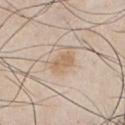<record>
<biopsy_status>not biopsied; imaged during a skin examination</biopsy_status>
<automated_metrics>
  <area_mm2_approx>6.0</area_mm2_approx>
  <eccentricity>0.8</eccentricity>
  <shape_asymmetry>0.2</shape_asymmetry>
  <cielab_L>64</cielab_L>
  <cielab_a>15</cielab_a>
  <cielab_b>32</cielab_b>
  <vs_skin_contrast_norm>6.5</vs_skin_contrast_norm>
  <nevus_likeness_0_100>45</nevus_likeness_0_100>
  <lesion_detection_confidence_0_100>100</lesion_detection_confidence_0_100>
</automated_metrics>
<patient>
  <sex>male</sex>
  <age_approx>45</age_approx>
</patient>
<image>
  <source>total-body photography crop</source>
  <field_of_view_mm>15</field_of_view_mm>
</image>
<site>front of the torso</site>
</record>